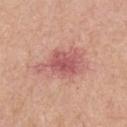The lesion was tiled from a total-body skin photograph and was not biopsied.
The recorded lesion diameter is about 6 mm.
From the arm.
Automated tile analysis of the lesion measured a lesion area of about 15 mm² and a shape-asymmetry score of about 0.4 (0 = symmetric). And it measured a border-irregularity index near 5.5/10, internal color variation of about 4 on a 0–10 scale, and peripheral color asymmetry of about 1. The analysis additionally found lesion-presence confidence of about 100/100.
A 15 mm close-up extracted from a 3D total-body photography capture.
A male subject, aged approximately 60.